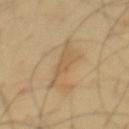Imaged during a routine full-body skin examination; the lesion was not biopsied and no histopathology is available. From the mid back. A lesion tile, about 15 mm wide, cut from a 3D total-body photograph. The patient is a male approximately 45 years of age. The tile uses cross-polarized illumination.